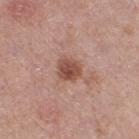workup: total-body-photography surveillance lesion; no biopsy
anatomic site: the right thigh
patient: female, about 40 years old
diameter: about 3 mm
tile lighting: white-light
imaging modality: 15 mm crop, total-body photography